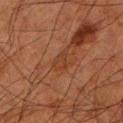<lesion>
<biopsy_status>not biopsied; imaged during a skin examination</biopsy_status>
<patient>
  <sex>male</sex>
  <age_approx>80</age_approx>
</patient>
<image>
  <source>total-body photography crop</source>
  <field_of_view_mm>15</field_of_view_mm>
</image>
<site>left lower leg</site>
<automated_metrics>
  <border_irregularity_0_10>4.0</border_irregularity_0_10>
  <color_variation_0_10>1.5</color_variation_0_10>
  <peripheral_color_asymmetry>0.5</peripheral_color_asymmetry>
</automated_metrics>
<lighting>cross-polarized</lighting>
</lesion>